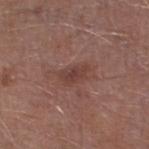notes = imaged on a skin check; not biopsied | subject = male, approximately 70 years of age | image = 15 mm crop, total-body photography | lesion size = about 3 mm | illumination = white-light illumination | anatomic site = the left lower leg.